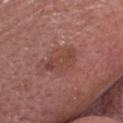Impression: Imaged during a routine full-body skin examination; the lesion was not biopsied and no histopathology is available. Context: The lesion is on the head or neck. The patient is a female roughly 80 years of age. A 15 mm crop from a total-body photograph taken for skin-cancer surveillance.